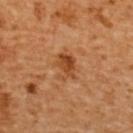An algorithmic analysis of the crop reported a lesion color around L≈48 a*≈26 b*≈41 in CIELAB, a lesion–skin lightness drop of about 10, and a lesion-to-skin contrast of about 8 (normalized; higher = more distinct). It also reported radial color variation of about 1.5. The lesion is on the upper back. Imaged with cross-polarized lighting. A roughly 15 mm field-of-view crop from a total-body skin photograph. The patient is a female roughly 55 years of age.